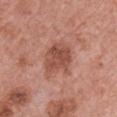A female patient aged 58 to 62. Captured under white-light illumination. Located on the chest. Cropped from a whole-body photographic skin survey; the tile spans about 15 mm.